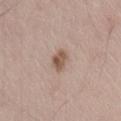Part of a total-body skin-imaging series; this lesion was reviewed on a skin check and was not flagged for biopsy.
A male patient, aged around 55.
The lesion is located on the lower back.
The lesion's longest dimension is about 2.5 mm.
A close-up tile cropped from a whole-body skin photograph, about 15 mm across.